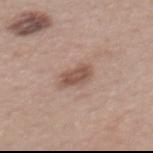No biopsy was performed on this lesion — it was imaged during a full skin examination and was not determined to be concerning.
On the mid back.
A female patient, about 55 years old.
A close-up tile cropped from a whole-body skin photograph, about 15 mm across.
This is a white-light tile.